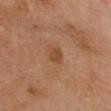| key | value |
|---|---|
| biopsy status | total-body-photography surveillance lesion; no biopsy |
| location | the head or neck |
| lighting | cross-polarized illumination |
| acquisition | total-body-photography crop, ~15 mm field of view |
| subject | male, aged approximately 60 |
| automated metrics | an eccentricity of roughly 0.8 and two-axis asymmetry of about 0.2; a classifier nevus-likeness of about 35/100 and a detector confidence of about 100 out of 100 that the crop contains a lesion |
| diameter | ~2.5 mm (longest diameter) |